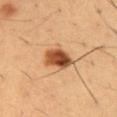{
  "biopsy_status": "not biopsied; imaged during a skin examination",
  "site": "abdomen",
  "image": {
    "source": "total-body photography crop",
    "field_of_view_mm": 15
  },
  "patient": {
    "sex": "male",
    "age_approx": 50
  }
}